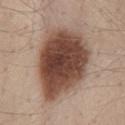Captured during whole-body skin photography for melanoma surveillance; the lesion was not biopsied.
Cropped from a whole-body photographic skin survey; the tile spans about 15 mm.
Approximately 9.5 mm at its widest.
Imaged with white-light lighting.
On the lower back.
A male patient, aged around 45.
The lesion-visualizer software estimated a shape eccentricity near 0.75 and a symmetry-axis asymmetry near 0.2. The analysis additionally found a lesion color around L≈45 a*≈19 b*≈26 in CIELAB, about 20 CIELAB-L* units darker than the surrounding skin, and a normalized border contrast of about 13.5.